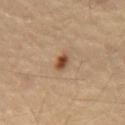Case summary:
• workup · catalogued during a skin exam; not biopsied
• lighting · cross-polarized illumination
• acquisition · ~15 mm crop, total-body skin-cancer survey
• TBP lesion metrics · an automated nevus-likeness rating near 95 out of 100
• size · ~2.5 mm (longest diameter)
• subject · male, roughly 55 years of age
• body site · the mid back